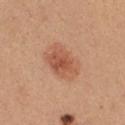The lesion was photographed on a routine skin check and not biopsied; there is no pathology result.
A female subject, aged approximately 25.
From the front of the torso.
A lesion tile, about 15 mm wide, cut from a 3D total-body photograph.